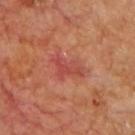No biopsy was performed on this lesion — it was imaged during a full skin examination and was not determined to be concerning.
A male subject in their mid-60s.
The recorded lesion diameter is about 4 mm.
The lesion is located on the chest.
A roughly 15 mm field-of-view crop from a total-body skin photograph.